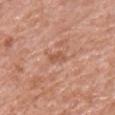Q: What are the patient's age and sex?
A: male, aged approximately 60
Q: What is the imaging modality?
A: 15 mm crop, total-body photography
Q: Lesion location?
A: the chest